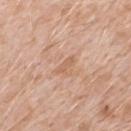Notes:
* workup — imaged on a skin check; not biopsied
* body site — the upper back
* image — total-body-photography crop, ~15 mm field of view
* size — ≈2.5 mm
* lighting — white-light
* automated metrics — a shape eccentricity near 0.9 and two-axis asymmetry of about 0.3
* subject — male, approximately 50 years of age